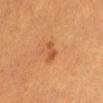Imaged during a routine full-body skin examination; the lesion was not biopsied and no histopathology is available.
A lesion tile, about 15 mm wide, cut from a 3D total-body photograph.
From the abdomen.
A female patient aged 28–32.